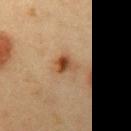This lesion was catalogued during total-body skin photography and was not selected for biopsy.
Captured under cross-polarized illumination.
This image is a 15 mm lesion crop taken from a total-body photograph.
On the left upper arm.
The subject is a male about 40 years old.
The recorded lesion diameter is about 3 mm.